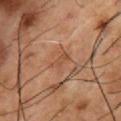Impression:
Recorded during total-body skin imaging; not selected for excision or biopsy.
Clinical summary:
A male patient, aged approximately 55. Imaged with cross-polarized lighting. Longest diameter approximately 3 mm. Cropped from a whole-body photographic skin survey; the tile spans about 15 mm. The lesion is located on the chest.